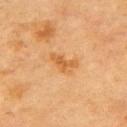Q: Was a biopsy performed?
A: imaged on a skin check; not biopsied
Q: What lighting was used for the tile?
A: cross-polarized illumination
Q: How large is the lesion?
A: ≈4 mm
Q: What are the patient's age and sex?
A: male, in their mid-80s or older
Q: Automated lesion metrics?
A: an area of roughly 5.5 mm², an eccentricity of roughly 0.9, and a symmetry-axis asymmetry near 0.35; a lesion color around L≈53 a*≈22 b*≈40 in CIELAB, roughly 8 lightness units darker than nearby skin, and a lesion-to-skin contrast of about 6.5 (normalized; higher = more distinct); internal color variation of about 2.5 on a 0–10 scale; a nevus-likeness score of about 5/100
Q: How was this image acquired?
A: ~15 mm crop, total-body skin-cancer survey
Q: What is the anatomic site?
A: the chest